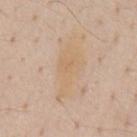The lesion was photographed on a routine skin check and not biopsied; there is no pathology result. The lesion is located on the front of the torso. A 15 mm crop from a total-body photograph taken for skin-cancer surveillance. A male subject, in their mid-50s.